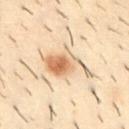workup: total-body-photography surveillance lesion; no biopsy | image: ~15 mm crop, total-body skin-cancer survey | subject: male, aged 28–32 | body site: the abdomen | lighting: cross-polarized illumination | size: about 5.5 mm | image-analysis metrics: an average lesion color of about L≈57 a*≈14 b*≈30 (CIELAB), roughly 10 lightness units darker than nearby skin, and a lesion-to-skin contrast of about 7.5 (normalized; higher = more distinct); border irregularity of about 4.5 on a 0–10 scale, internal color variation of about 8.5 on a 0–10 scale, and radial color variation of about 2.5.